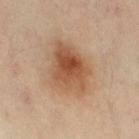Part of a total-body skin-imaging series; this lesion was reviewed on a skin check and was not flagged for biopsy. The tile uses cross-polarized illumination. The lesion is located on the leg. The subject is a female about 40 years old. A region of skin cropped from a whole-body photographic capture, roughly 15 mm wide. The recorded lesion diameter is about 6 mm.